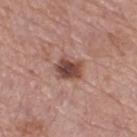workup — no biopsy performed (imaged during a skin exam) | image — ~15 mm tile from a whole-body skin photo | patient — female, approximately 55 years of age | illumination — white-light | image-analysis metrics — a footprint of about 7.5 mm², an eccentricity of roughly 0.55, and a symmetry-axis asymmetry near 0.25; border irregularity of about 2.5 on a 0–10 scale, a color-variation rating of about 5/10, and a peripheral color-asymmetry measure near 1.5; a classifier nevus-likeness of about 75/100 and lesion-presence confidence of about 100/100 | site — the right thigh | size — ~3.5 mm (longest diameter).